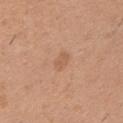Clinical impression:
Imaged during a routine full-body skin examination; the lesion was not biopsied and no histopathology is available.
Image and clinical context:
This is a white-light tile. The lesion is located on the left upper arm. The recorded lesion diameter is about 2.5 mm. This image is a 15 mm lesion crop taken from a total-body photograph. The patient is a male approximately 40 years of age.